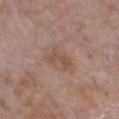This lesion was catalogued during total-body skin photography and was not selected for biopsy.
Captured under white-light illumination.
The lesion-visualizer software estimated a footprint of about 4 mm², an eccentricity of roughly 0.85, and a shape-asymmetry score of about 0.4 (0 = symmetric). And it measured a mean CIELAB color near L≈50 a*≈19 b*≈26, roughly 7 lightness units darker than nearby skin, and a lesion-to-skin contrast of about 5.5 (normalized; higher = more distinct). The software also gave border irregularity of about 5 on a 0–10 scale, a color-variation rating of about 1/10, and a peripheral color-asymmetry measure near 0.
From the front of the torso.
A 15 mm crop from a total-body photograph taken for skin-cancer surveillance.
A female patient, aged 83 or older.
Longest diameter approximately 3 mm.